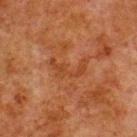The lesion was photographed on a routine skin check and not biopsied; there is no pathology result. The tile uses cross-polarized illumination. Automated tile analysis of the lesion measured internal color variation of about 0.5 on a 0–10 scale and peripheral color asymmetry of about 0. It also reported a nevus-likeness score of about 0/100 and a detector confidence of about 100 out of 100 that the crop contains a lesion. This image is a 15 mm lesion crop taken from a total-body photograph. A male patient, aged 78–82. The recorded lesion diameter is about 4 mm. The lesion is located on the upper back.